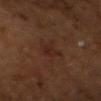<lesion>
  <image>
    <source>total-body photography crop</source>
    <field_of_view_mm>15</field_of_view_mm>
  </image>
  <patient>
    <sex>male</sex>
    <age_approx>55</age_approx>
  </patient>
  <lighting>cross-polarized</lighting>
  <lesion_size>
    <long_diameter_mm_approx>3.0</long_diameter_mm_approx>
  </lesion_size>
  <site>head or neck</site>
</lesion>